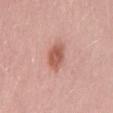Approximately 3.5 mm at its widest.
A male patient, approximately 55 years of age.
This image is a 15 mm lesion crop taken from a total-body photograph.
The lesion is located on the back.
Captured under white-light illumination.